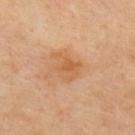notes = total-body-photography surveillance lesion; no biopsy